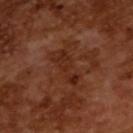This lesion was catalogued during total-body skin photography and was not selected for biopsy.
A male subject, aged 63–67.
A roughly 15 mm field-of-view crop from a total-body skin photograph.